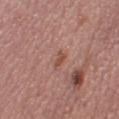This lesion was catalogued during total-body skin photography and was not selected for biopsy.
On the left thigh.
A 15 mm close-up tile from a total-body photography series done for melanoma screening.
Imaged with white-light lighting.
The patient is a female aged around 50.